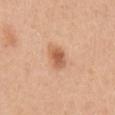The lesion was photographed on a routine skin check and not biopsied; there is no pathology result.
Longest diameter approximately 3 mm.
A lesion tile, about 15 mm wide, cut from a 3D total-body photograph.
A female subject roughly 45 years of age.
On the left upper arm.